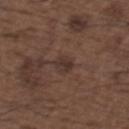Captured during whole-body skin photography for melanoma surveillance; the lesion was not biopsied. Approximately 2.5 mm at its widest. From the upper back. A 15 mm crop from a total-body photograph taken for skin-cancer surveillance. The patient is a male in their 50s. Imaged with white-light lighting. Automated tile analysis of the lesion measured an average lesion color of about L≈33 a*≈16 b*≈20 (CIELAB), a lesion–skin lightness drop of about 6, and a normalized border contrast of about 7. The analysis additionally found a within-lesion color-variation index near 2/10 and radial color variation of about 1. The analysis additionally found a detector confidence of about 100 out of 100 that the crop contains a lesion.